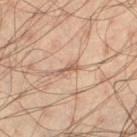The lesion was photographed on a routine skin check and not biopsied; there is no pathology result. Captured under cross-polarized illumination. From the left thigh. A roughly 15 mm field-of-view crop from a total-body skin photograph. The recorded lesion diameter is about 3 mm. A male patient, aged around 35.